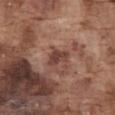Q: Is there a histopathology result?
A: no biopsy performed (imaged during a skin exam)
Q: Where on the body is the lesion?
A: the abdomen
Q: How was this image acquired?
A: ~15 mm crop, total-body skin-cancer survey
Q: Who is the patient?
A: male, in their mid-70s
Q: Illumination type?
A: white-light
Q: How large is the lesion?
A: ≈3 mm